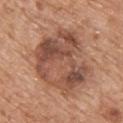Assessment: Captured during whole-body skin photography for melanoma surveillance; the lesion was not biopsied. Image and clinical context: A male subject, approximately 60 years of age. From the upper back. The tile uses white-light illumination. Measured at roughly 8.5 mm in maximum diameter. Automated image analysis of the tile measured a border-irregularity rating of about 4/10, a color-variation rating of about 8.5/10, and peripheral color asymmetry of about 3. The software also gave an automated nevus-likeness rating near 0 out of 100 and lesion-presence confidence of about 100/100. Cropped from a total-body skin-imaging series; the visible field is about 15 mm.